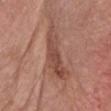Q: Was a biopsy performed?
A: catalogued during a skin exam; not biopsied
Q: What is the anatomic site?
A: the head or neck
Q: What are the patient's age and sex?
A: male, aged approximately 80
Q: What kind of image is this?
A: ~15 mm tile from a whole-body skin photo
Q: How was the tile lit?
A: white-light illumination
Q: Lesion size?
A: ≈7.5 mm